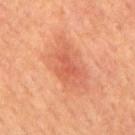– notes — imaged on a skin check; not biopsied
– image source — ~15 mm crop, total-body skin-cancer survey
– lighting — cross-polarized
– site — the back
– subject — male, aged 63 to 67
– automated lesion analysis — a lesion color around L≈51 a*≈30 b*≈33 in CIELAB, a lesion–skin lightness drop of about 7, and a normalized border contrast of about 5; a classifier nevus-likeness of about 80/100 and a lesion-detection confidence of about 100/100
– size — ≈3 mm